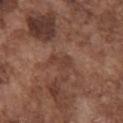biopsy status=total-body-photography surveillance lesion; no biopsy | lesion diameter=≈3 mm | subject=male, aged around 75 | acquisition=~15 mm crop, total-body skin-cancer survey | anatomic site=the chest | tile lighting=white-light illumination.